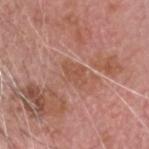The lesion was tiled from a total-body skin photograph and was not biopsied.
A male patient aged 73–77.
On the head or neck.
Imaged with white-light lighting.
Automated image analysis of the tile measured an area of roughly 5 mm², a shape eccentricity near 0.75, and a symmetry-axis asymmetry near 0.2. The analysis additionally found a nevus-likeness score of about 0/100 and a detector confidence of about 100 out of 100 that the crop contains a lesion.
This image is a 15 mm lesion crop taken from a total-body photograph.
Measured at roughly 3 mm in maximum diameter.